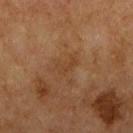Clinical impression:
Imaged during a routine full-body skin examination; the lesion was not biopsied and no histopathology is available.
Clinical summary:
About 2.5 mm across. Imaged with cross-polarized lighting. A 15 mm close-up tile from a total-body photography series done for melanoma screening. The lesion is located on the right upper arm. Automated tile analysis of the lesion measured an average lesion color of about L≈36 a*≈18 b*≈30 (CIELAB), about 5 CIELAB-L* units darker than the surrounding skin, and a normalized border contrast of about 5. And it measured a border-irregularity index near 4.5/10 and internal color variation of about 0.5 on a 0–10 scale. The patient is a female approximately 60 years of age.